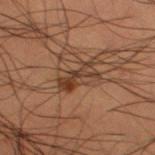Q: Was this lesion biopsied?
A: catalogued during a skin exam; not biopsied
Q: What lighting was used for the tile?
A: cross-polarized
Q: Patient demographics?
A: male, aged around 50
Q: What kind of image is this?
A: total-body-photography crop, ~15 mm field of view
Q: How large is the lesion?
A: about 4 mm
Q: What is the anatomic site?
A: the right thigh
Q: Automated lesion metrics?
A: a footprint of about 6 mm², an eccentricity of roughly 0.9, and a symmetry-axis asymmetry near 0.35; an average lesion color of about L≈30 a*≈16 b*≈24 (CIELAB), a lesion–skin lightness drop of about 8, and a lesion-to-skin contrast of about 8 (normalized; higher = more distinct); an automated nevus-likeness rating near 20 out of 100